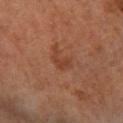| feature | finding |
|---|---|
| workup | no biopsy performed (imaged during a skin exam) |
| image | 15 mm crop, total-body photography |
| location | the right lower leg |
| tile lighting | cross-polarized illumination |
| size | ~2.5 mm (longest diameter) |
| subject | female, aged 63–67 |
| automated metrics | a border-irregularity rating of about 5.5/10, a within-lesion color-variation index near 1/10, and radial color variation of about 0.5; a nevus-likeness score of about 15/100 and a detector confidence of about 100 out of 100 that the crop contains a lesion |